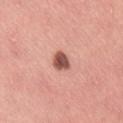Q: Was this lesion biopsied?
A: no biopsy performed (imaged during a skin exam)
Q: How was the tile lit?
A: white-light illumination
Q: Patient demographics?
A: female, aged 28 to 32
Q: What kind of image is this?
A: ~15 mm tile from a whole-body skin photo
Q: What is the anatomic site?
A: the right thigh